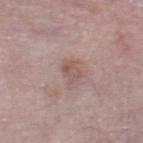notes: total-body-photography surveillance lesion; no biopsy
automated lesion analysis: a lesion color around L≈54 a*≈18 b*≈23 in CIELAB and a lesion-to-skin contrast of about 6 (normalized; higher = more distinct); an automated nevus-likeness rating near 5 out of 100 and a lesion-detection confidence of about 100/100
subject: male, aged 73 to 77
site: the right thigh
size: about 2.5 mm
imaging modality: total-body-photography crop, ~15 mm field of view